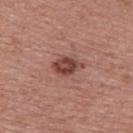workup: catalogued during a skin exam; not biopsied
body site: the upper back
subject: male, in their mid-50s
size: about 3.5 mm
image: ~15 mm crop, total-body skin-cancer survey
automated metrics: an average lesion color of about L≈44 a*≈24 b*≈24 (CIELAB), roughly 13 lightness units darker than nearby skin, and a lesion-to-skin contrast of about 9.5 (normalized; higher = more distinct); a classifier nevus-likeness of about 70/100